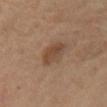This is a cross-polarized tile.
The lesion is located on the right lower leg.
The recorded lesion diameter is about 3.5 mm.
A 15 mm crop from a total-body photograph taken for skin-cancer surveillance.
A female subject, about 65 years old.